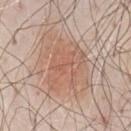Assessment: Captured during whole-body skin photography for melanoma surveillance; the lesion was not biopsied. Image and clinical context: Automated tile analysis of the lesion measured an area of roughly 20 mm² and a shape eccentricity near 0.65. The analysis additionally found a border-irregularity index near 4/10, a color-variation rating of about 3.5/10, and a peripheral color-asymmetry measure near 1. It also reported a classifier nevus-likeness of about 100/100 and a detector confidence of about 90 out of 100 that the crop contains a lesion. A 15 mm crop from a total-body photograph taken for skin-cancer surveillance. Located on the chest. Imaged with white-light lighting. A male subject, aged around 80. Longest diameter approximately 6 mm.